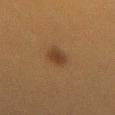The lesion was photographed on a routine skin check and not biopsied; there is no pathology result. This is a cross-polarized tile. A 15 mm crop from a total-body photograph taken for skin-cancer surveillance. A female subject, aged 38 to 42. From the lower back. Approximately 3.5 mm at its widest. The total-body-photography lesion software estimated a color-variation rating of about 1.5/10.